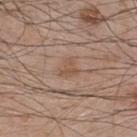Clinical impression: Imaged during a routine full-body skin examination; the lesion was not biopsied and no histopathology is available. Acquisition and patient details: Located on the upper back. A 15 mm close-up tile from a total-body photography series done for melanoma screening. A male subject, aged 48 to 52.